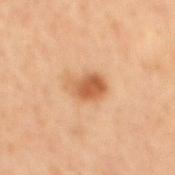| key | value |
|---|---|
| biopsy status | catalogued during a skin exam; not biopsied |
| patient | male, aged 58–62 |
| diameter | about 4 mm |
| imaging modality | 15 mm crop, total-body photography |
| site | the mid back |
| tile lighting | cross-polarized |